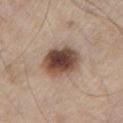Case summary:
* lighting: white-light illumination
* automated lesion analysis: a lesion area of about 15 mm², a shape eccentricity near 0.55, and a shape-asymmetry score of about 0.1 (0 = symmetric); internal color variation of about 8 on a 0–10 scale; an automated nevus-likeness rating near 100 out of 100 and a detector confidence of about 100 out of 100 that the crop contains a lesion
* body site: the chest
* subject: male, about 80 years old
* lesion diameter: ≈5 mm
* imaging modality: total-body-photography crop, ~15 mm field of view